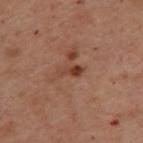Findings:
- follow-up: catalogued during a skin exam; not biopsied
- patient: female, in their mid-50s
- imaging modality: total-body-photography crop, ~15 mm field of view
- image-analysis metrics: a footprint of about 3 mm², an eccentricity of roughly 0.9, and a shape-asymmetry score of about 0.35 (0 = symmetric); a lesion color around L≈33 a*≈20 b*≈25 in CIELAB and a normalized border contrast of about 8; a detector confidence of about 100 out of 100 that the crop contains a lesion
- lighting: cross-polarized illumination
- site: the back